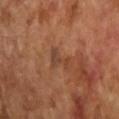Impression:
No biopsy was performed on this lesion — it was imaged during a full skin examination and was not determined to be concerning.
Context:
A female patient, aged approximately 70. Captured under cross-polarized illumination. The lesion is located on the arm. The total-body-photography lesion software estimated border irregularity of about 6.5 on a 0–10 scale, a within-lesion color-variation index near 1/10, and radial color variation of about 0.5. And it measured an automated nevus-likeness rating near 0 out of 100 and lesion-presence confidence of about 100/100. The lesion's longest dimension is about 3 mm. A region of skin cropped from a whole-body photographic capture, roughly 15 mm wide.